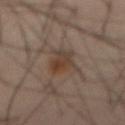<record>
<biopsy_status>not biopsied; imaged during a skin examination</biopsy_status>
<image>
  <source>total-body photography crop</source>
  <field_of_view_mm>15</field_of_view_mm>
</image>
<lesion_size>
  <long_diameter_mm_approx>4.5</long_diameter_mm_approx>
</lesion_size>
<lighting>cross-polarized</lighting>
<patient>
  <sex>male</sex>
  <age_approx>60</age_approx>
</patient>
<site>abdomen</site>
</record>